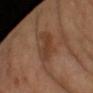Case summary:
* biopsy status: catalogued during a skin exam; not biopsied
* acquisition: total-body-photography crop, ~15 mm field of view
* patient: male, approximately 65 years of age
* illumination: cross-polarized illumination